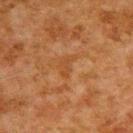Q: What are the patient's age and sex?
A: male, aged around 80
Q: What kind of image is this?
A: ~15 mm crop, total-body skin-cancer survey
Q: What is the lesion's diameter?
A: ~2.5 mm (longest diameter)
Q: How was the tile lit?
A: cross-polarized illumination
Q: Where on the body is the lesion?
A: the upper back
Q: Automated lesion metrics?
A: an automated nevus-likeness rating near 0 out of 100 and a detector confidence of about 100 out of 100 that the crop contains a lesion